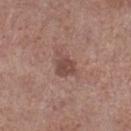Case summary:
- notes · total-body-photography surveillance lesion; no biopsy
- image · ~15 mm crop, total-body skin-cancer survey
- patient · male, roughly 55 years of age
- body site · the leg
- tile lighting · white-light illumination
- diameter · about 2.5 mm